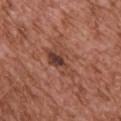This lesion was catalogued during total-body skin photography and was not selected for biopsy.
A lesion tile, about 15 mm wide, cut from a 3D total-body photograph.
A male patient, aged 73 to 77.
The lesion is on the upper back.